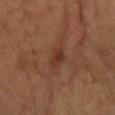acquisition — ~15 mm tile from a whole-body skin photo | location — the right forearm | lighting — cross-polarized illumination | patient — female, aged 58–62 | lesion diameter — ~2.5 mm (longest diameter).